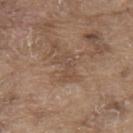Assessment:
No biopsy was performed on this lesion — it was imaged during a full skin examination and was not determined to be concerning.
Context:
The lesion is located on the upper back. The tile uses white-light illumination. A roughly 15 mm field-of-view crop from a total-body skin photograph. Automated tile analysis of the lesion measured border irregularity of about 5.5 on a 0–10 scale, internal color variation of about 3.5 on a 0–10 scale, and peripheral color asymmetry of about 1.5. And it measured a classifier nevus-likeness of about 0/100 and a lesion-detection confidence of about 95/100. A male patient aged around 80. Measured at roughly 3 mm in maximum diameter.